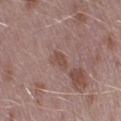A male subject, aged approximately 40.
Cropped from a total-body skin-imaging series; the visible field is about 15 mm.
On the leg.